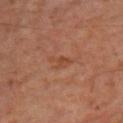Q: What kind of image is this?
A: ~15 mm tile from a whole-body skin photo
Q: Who is the patient?
A: male, roughly 60 years of age
Q: Lesion size?
A: ≈2.5 mm
Q: Where on the body is the lesion?
A: the right lower leg